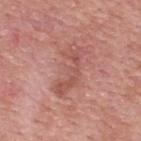Impression: Recorded during total-body skin imaging; not selected for excision or biopsy. Context: A roughly 15 mm field-of-view crop from a total-body skin photograph. The total-body-photography lesion software estimated a footprint of about 11 mm² and two-axis asymmetry of about 0.5. It also reported a lesion color around L≈54 a*≈26 b*≈26 in CIELAB and a lesion-to-skin contrast of about 5.5 (normalized; higher = more distinct). The software also gave a border-irregularity rating of about 7.5/10 and a color-variation rating of about 3/10. It also reported an automated nevus-likeness rating near 0 out of 100 and lesion-presence confidence of about 100/100. Captured under white-light illumination. A female patient aged 38–42. The lesion is located on the upper back. Approximately 6 mm at its widest.